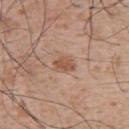notes = catalogued during a skin exam; not biopsied | imaging modality = total-body-photography crop, ~15 mm field of view | subject = male, approximately 65 years of age | body site = the upper back | lighting = white-light illumination.